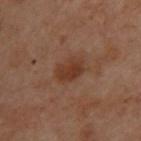| key | value |
|---|---|
| notes | no biopsy performed (imaged during a skin exam) |
| image source | ~15 mm tile from a whole-body skin photo |
| lesion diameter | ~3.5 mm (longest diameter) |
| subject | female, approximately 55 years of age |
| body site | the upper back |
| automated lesion analysis | an area of roughly 6.5 mm², an eccentricity of roughly 0.75, and a symmetry-axis asymmetry near 0.15; a border-irregularity index near 1.5/10 and a color-variation rating of about 2/10; a lesion-detection confidence of about 100/100 |